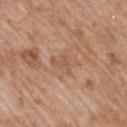| feature | finding |
|---|---|
| biopsy status | no biopsy performed (imaged during a skin exam) |
| illumination | white-light illumination |
| imaging modality | ~15 mm crop, total-body skin-cancer survey |
| size | ≈3 mm |
| body site | the mid back |
| subject | male, aged approximately 60 |
| automated lesion analysis | a border-irregularity rating of about 7.5/10, a within-lesion color-variation index near 0/10, and radial color variation of about 0; a nevus-likeness score of about 0/100 and a detector confidence of about 100 out of 100 that the crop contains a lesion |